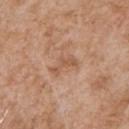Image and clinical context:
A close-up tile cropped from a whole-body skin photograph, about 15 mm across. The lesion is located on the front of the torso. A male patient aged 63 to 67.